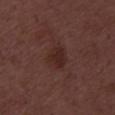Clinical impression:
Part of a total-body skin-imaging series; this lesion was reviewed on a skin check and was not flagged for biopsy.
Acquisition and patient details:
A male patient, aged 48 to 52. An algorithmic analysis of the crop reported a classifier nevus-likeness of about 45/100 and a lesion-detection confidence of about 100/100. Captured under white-light illumination. Cropped from a whole-body photographic skin survey; the tile spans about 15 mm.